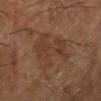Notes:
– body site · the leg
– imaging modality · ~15 mm crop, total-body skin-cancer survey
– subject · male, aged around 65
– lesion diameter · about 6.5 mm
– illumination · cross-polarized illumination